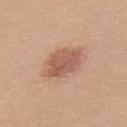Clinical impression: Part of a total-body skin-imaging series; this lesion was reviewed on a skin check and was not flagged for biopsy. Acquisition and patient details: The subject is a female approximately 20 years of age. This image is a 15 mm lesion crop taken from a total-body photograph. On the upper back. This is a white-light tile. The lesion's longest dimension is about 5 mm.